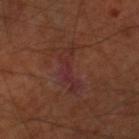<lesion>
  <image>
    <source>total-body photography crop</source>
    <field_of_view_mm>15</field_of_view_mm>
  </image>
  <patient>
    <sex>male</sex>
    <age_approx>70</age_approx>
  </patient>
  <site>right thigh</site>
  <lesion_size>
    <long_diameter_mm_approx>5.5</long_diameter_mm_approx>
  </lesion_size>
</lesion>